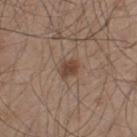<case>
  <biopsy_status>not biopsied; imaged during a skin examination</biopsy_status>
</case>